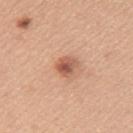<case>
<biopsy_status>not biopsied; imaged during a skin examination</biopsy_status>
<patient>
  <sex>female</sex>
  <age_approx>40</age_approx>
</patient>
<lesion_size>
  <long_diameter_mm_approx>2.5</long_diameter_mm_approx>
</lesion_size>
<image>
  <source>total-body photography crop</source>
  <field_of_view_mm>15</field_of_view_mm>
</image>
<site>left upper arm</site>
<lighting>white-light</lighting>
<automated_metrics>
  <vs_skin_darker_L>13.0</vs_skin_darker_L>
  <vs_skin_contrast_norm>8.5</vs_skin_contrast_norm>
</automated_metrics>
</case>